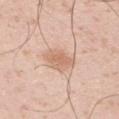<case>
<biopsy_status>not biopsied; imaged during a skin examination</biopsy_status>
<lesion_size>
  <long_diameter_mm_approx>3.0</long_diameter_mm_approx>
</lesion_size>
<patient>
  <sex>male</sex>
  <age_approx>40</age_approx>
</patient>
<site>left upper arm</site>
<image>
  <source>total-body photography crop</source>
  <field_of_view_mm>15</field_of_view_mm>
</image>
</case>